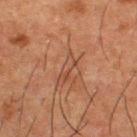Assessment: This lesion was catalogued during total-body skin photography and was not selected for biopsy. Context: A roughly 15 mm field-of-view crop from a total-body skin photograph. Located on the back. A male subject, aged 48–52.